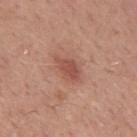| key | value |
|---|---|
| image source | ~15 mm crop, total-body skin-cancer survey |
| location | the mid back |
| subject | male, aged approximately 50 |
| TBP lesion metrics | a shape eccentricity near 0.8 and a symmetry-axis asymmetry near 0.25; a mean CIELAB color near L≈51 a*≈26 b*≈27, about 9 CIELAB-L* units darker than the surrounding skin, and a lesion-to-skin contrast of about 6.5 (normalized; higher = more distinct); a classifier nevus-likeness of about 20/100 and lesion-presence confidence of about 100/100 |